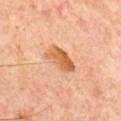The lesion was photographed on a routine skin check and not biopsied; there is no pathology result. A close-up tile cropped from a whole-body skin photograph, about 15 mm across. The lesion is located on the chest. The lesion's longest dimension is about 4.5 mm. A male patient, about 70 years old.